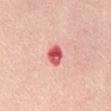Recorded during total-body skin imaging; not selected for excision or biopsy. A 15 mm close-up extracted from a 3D total-body photography capture. Captured under white-light illumination. The lesion is located on the abdomen. The patient is a female aged 58 to 62. About 3 mm across. The lesion-visualizer software estimated a border-irregularity rating of about 1.5/10, a color-variation rating of about 8/10, and radial color variation of about 2.5. The analysis additionally found a classifier nevus-likeness of about 0/100 and lesion-presence confidence of about 100/100.